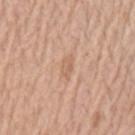A male patient, aged 63 to 67. A roughly 15 mm field-of-view crop from a total-body skin photograph. On the left upper arm.